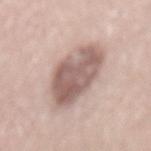Impression:
The lesion was tiled from a total-body skin photograph and was not biopsied.
Background:
The lesion is located on the mid back. A male patient about 60 years old. This image is a 15 mm lesion crop taken from a total-body photograph. Measured at roughly 6.5 mm in maximum diameter.